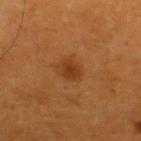site: the upper back; subject: male, aged 58–62; lesion size: about 2.5 mm; tile lighting: cross-polarized; imaging modality: ~15 mm crop, total-body skin-cancer survey.